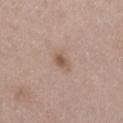{"image": {"source": "total-body photography crop", "field_of_view_mm": 15}, "patient": {"sex": "male", "age_approx": 50}, "lesion_size": {"long_diameter_mm_approx": 2.5}, "site": "lower back"}